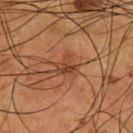• notes · total-body-photography surveillance lesion; no biopsy
• subject · male, about 55 years old
• acquisition · ~15 mm crop, total-body skin-cancer survey
• lighting · cross-polarized
• anatomic site · the chest